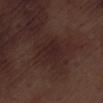| key | value |
|---|---|
| follow-up | no biopsy performed (imaged during a skin exam) |
| subject | male, roughly 70 years of age |
| body site | the left lower leg |
| lesion diameter | about 3.5 mm |
| illumination | white-light |
| image source | total-body-photography crop, ~15 mm field of view |